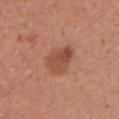Q: Was a biopsy performed?
A: imaged on a skin check; not biopsied
Q: What are the patient's age and sex?
A: female, roughly 25 years of age
Q: What is the anatomic site?
A: the left upper arm
Q: What is the imaging modality?
A: 15 mm crop, total-body photography